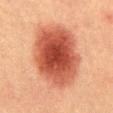Image and clinical context: The subject is a male aged around 40. Measured at roughly 8.5 mm in maximum diameter. From the abdomen. A lesion tile, about 15 mm wide, cut from a 3D total-body photograph. The tile uses cross-polarized illumination.